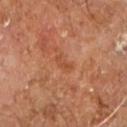<tbp_lesion>
  <biopsy_status>not biopsied; imaged during a skin examination</biopsy_status>
  <patient>
    <sex>male</sex>
    <age_approx>60</age_approx>
  </patient>
  <site>right leg</site>
  <lighting>cross-polarized</lighting>
  <image>
    <source>total-body photography crop</source>
    <field_of_view_mm>15</field_of_view_mm>
  </image>
  <lesion_size>
    <long_diameter_mm_approx>2.5</long_diameter_mm_approx>
  </lesion_size>
  <automated_metrics>
    <nevus_likeness_0_100>0</nevus_likeness_0_100>
    <lesion_detection_confidence_0_100>100</lesion_detection_confidence_0_100>
  </automated_metrics>
</tbp_lesion>